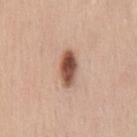  biopsy_status: not biopsied; imaged during a skin examination
  patient:
    sex: male
    age_approx: 40
  image:
    source: total-body photography crop
    field_of_view_mm: 15
  automated_metrics:
    area_mm2_approx: 7.5
    eccentricity: 0.9
    shape_asymmetry: 0.15
  site: chest